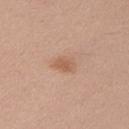The lesion was photographed on a routine skin check and not biopsied; there is no pathology result. A female subject, approximately 35 years of age. This image is a 15 mm lesion crop taken from a total-body photograph. From the left upper arm.